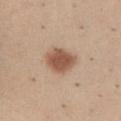Impression:
Captured during whole-body skin photography for melanoma surveillance; the lesion was not biopsied.
Image and clinical context:
A close-up tile cropped from a whole-body skin photograph, about 15 mm across. The tile uses white-light illumination. On the abdomen. Approximately 3.5 mm at its widest. A male subject, roughly 35 years of age. An algorithmic analysis of the crop reported a border-irregularity rating of about 1.5/10 and internal color variation of about 3 on a 0–10 scale.